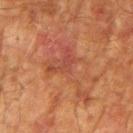Assessment:
Captured during whole-body skin photography for melanoma surveillance; the lesion was not biopsied.
Acquisition and patient details:
Cropped from a whole-body photographic skin survey; the tile spans about 15 mm. Captured under cross-polarized illumination. A male patient aged around 75. Automated image analysis of the tile measured a footprint of about 17 mm² and a shape eccentricity near 0.85. Located on the right upper arm. The recorded lesion diameter is about 8 mm.